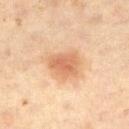This lesion was catalogued during total-body skin photography and was not selected for biopsy. This image is a 15 mm lesion crop taken from a total-body photograph. The lesion's longest dimension is about 4.5 mm. A male subject, aged around 60. The lesion is located on the right thigh.